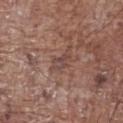Case summary:
• biopsy status: no biopsy performed (imaged during a skin exam)
• lesion size: ~2.5 mm (longest diameter)
• location: the abdomen
• imaging modality: ~15 mm tile from a whole-body skin photo
• TBP lesion metrics: a lesion area of about 3.5 mm², an outline eccentricity of about 0.8 (0 = round, 1 = elongated), and a shape-asymmetry score of about 0.3 (0 = symmetric); a mean CIELAB color near L≈45 a*≈19 b*≈22, about 7 CIELAB-L* units darker than the surrounding skin, and a lesion-to-skin contrast of about 6 (normalized; higher = more distinct); a classifier nevus-likeness of about 0/100 and lesion-presence confidence of about 95/100
• illumination: white-light illumination
• patient: male, roughly 70 years of age